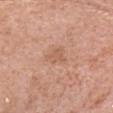Assessment:
This lesion was catalogued during total-body skin photography and was not selected for biopsy.
Clinical summary:
A female subject, roughly 50 years of age. The lesion is on the head or neck. This is a white-light tile. The total-body-photography lesion software estimated a mean CIELAB color near L≈59 a*≈22 b*≈32 and roughly 6 lightness units darker than nearby skin. The software also gave a border-irregularity rating of about 3.5/10, a within-lesion color-variation index near 2/10, and peripheral color asymmetry of about 1. And it measured a nevus-likeness score of about 0/100 and a lesion-detection confidence of about 100/100. A close-up tile cropped from a whole-body skin photograph, about 15 mm across.